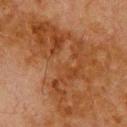Findings:
* notes — no biopsy performed (imaged during a skin exam)
* body site — the upper back
* tile lighting — cross-polarized
* size — about 13 mm
* patient — male, aged 78 to 82
* imaging modality — 15 mm crop, total-body photography